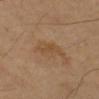The lesion was tiled from a total-body skin photograph and was not biopsied. The lesion's longest dimension is about 3.5 mm. The subject is a male in their mid- to late 60s. This image is a 15 mm lesion crop taken from a total-body photograph. The lesion is on the arm.